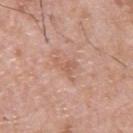diameter: about 2.5 mm
tile lighting: white-light
automated metrics: a nevus-likeness score of about 0/100
patient: male, in their mid- to late 50s
image: ~15 mm tile from a whole-body skin photo